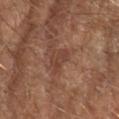  biopsy_status: not biopsied; imaged during a skin examination
  site: right upper arm
  patient:
    sex: male
    age_approx: 65
  image:
    source: total-body photography crop
    field_of_view_mm: 15
  lesion_size:
    long_diameter_mm_approx: 3.5